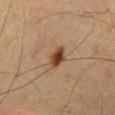Assessment: Part of a total-body skin-imaging series; this lesion was reviewed on a skin check and was not flagged for biopsy. Context: A male patient approximately 60 years of age. The lesion's longest dimension is about 2.5 mm. The total-body-photography lesion software estimated a footprint of about 5 mm² and an outline eccentricity of about 0.7 (0 = round, 1 = elongated). The software also gave border irregularity of about 2 on a 0–10 scale, a color-variation rating of about 4.5/10, and radial color variation of about 1.5. A 15 mm crop from a total-body photograph taken for skin-cancer surveillance. Imaged with cross-polarized lighting. From the mid back.